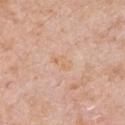• biopsy status: no biopsy performed (imaged during a skin exam)
• image-analysis metrics: a mean CIELAB color near L≈67 a*≈18 b*≈34, roughly 4 lightness units darker than nearby skin, and a normalized lesion–skin contrast near 5
• subject: male, about 75 years old
• imaging modality: ~15 mm crop, total-body skin-cancer survey
• lesion diameter: ~2.5 mm (longest diameter)
• body site: the right upper arm